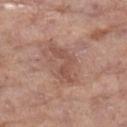{
  "biopsy_status": "not biopsied; imaged during a skin examination",
  "patient": {
    "sex": "female",
    "age_approx": 75
  },
  "lesion_size": {
    "long_diameter_mm_approx": 5.0
  },
  "image": {
    "source": "total-body photography crop",
    "field_of_view_mm": 15
  },
  "site": "leg"
}